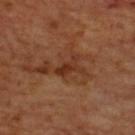Findings:
* follow-up · catalogued during a skin exam; not biopsied
* tile lighting · cross-polarized
* TBP lesion metrics · a lesion area of about 4 mm² and a symmetry-axis asymmetry near 0.5; a mean CIELAB color near L≈31 a*≈23 b*≈30, about 8 CIELAB-L* units darker than the surrounding skin, and a normalized lesion–skin contrast near 7.5; a border-irregularity rating of about 5/10, internal color variation of about 1.5 on a 0–10 scale, and radial color variation of about 0.5
* image · total-body-photography crop, ~15 mm field of view
* subject · male, about 70 years old
* lesion size · about 3 mm
* site · the upper back